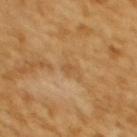{
  "biopsy_status": "not biopsied; imaged during a skin examination",
  "lesion_size": {
    "long_diameter_mm_approx": 1.5
  },
  "automated_metrics": {
    "eccentricity": 0.85,
    "shape_asymmetry": 0.4,
    "cielab_L": 53,
    "cielab_a": 20,
    "cielab_b": 40,
    "vs_skin_darker_L": 7.0,
    "vs_skin_contrast_norm": 5.0,
    "border_irregularity_0_10": 3.5,
    "nevus_likeness_0_100": 0,
    "lesion_detection_confidence_0_100": 100
  },
  "patient": {
    "sex": "female",
    "age_approx": 55
  },
  "image": {
    "source": "total-body photography crop",
    "field_of_view_mm": 15
  },
  "site": "back"
}